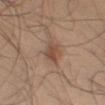Part of a total-body skin-imaging series; this lesion was reviewed on a skin check and was not flagged for biopsy. A male patient, aged 43–47. The total-body-photography lesion software estimated a lesion area of about 5.5 mm² and a shape-asymmetry score of about 0.25 (0 = symmetric). The analysis additionally found a mean CIELAB color near L≈49 a*≈19 b*≈28, a lesion–skin lightness drop of about 8, and a lesion-to-skin contrast of about 6.5 (normalized; higher = more distinct). A 15 mm crop from a total-body photograph taken for skin-cancer surveillance. From the right upper arm.